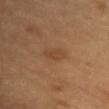The lesion is located on the chest. A 15 mm crop from a total-body photograph taken for skin-cancer surveillance. A female subject, aged 38–42.